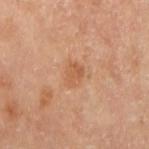{"biopsy_status": "not biopsied; imaged during a skin examination", "patient": {"sex": "female", "age_approx": 65}, "image": {"source": "total-body photography crop", "field_of_view_mm": 15}, "lighting": "cross-polarized", "site": "right upper arm", "automated_metrics": {"area_mm2_approx": 4.5, "eccentricity": 0.7, "cielab_L": 57, "cielab_a": 23, "cielab_b": 36, "vs_skin_contrast_norm": 5.5, "border_irregularity_0_10": 2.0, "color_variation_0_10": 2.0, "peripheral_color_asymmetry": 0.5}}